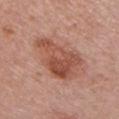{
  "biopsy_status": "not biopsied; imaged during a skin examination",
  "lighting": "white-light",
  "site": "chest",
  "automated_metrics": {
    "cielab_L": 51,
    "cielab_a": 25,
    "cielab_b": 29,
    "vs_skin_darker_L": 11.0,
    "vs_skin_contrast_norm": 8.0,
    "nevus_likeness_0_100": 75,
    "lesion_detection_confidence_0_100": 100
  },
  "lesion_size": {
    "long_diameter_mm_approx": 6.5
  },
  "patient": {
    "sex": "female",
    "age_approx": 50
  },
  "image": {
    "source": "total-body photography crop",
    "field_of_view_mm": 15
  }
}